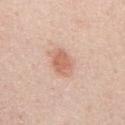The lesion was photographed on a routine skin check and not biopsied; there is no pathology result.